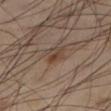{"biopsy_status": "not biopsied; imaged during a skin examination", "automated_metrics": {"area_mm2_approx": 2.5, "shape_asymmetry": 0.35, "nevus_likeness_0_100": 75, "lesion_detection_confidence_0_100": 100}, "image": {"source": "total-body photography crop", "field_of_view_mm": 15}, "patient": {"sex": "male", "age_approx": 55}, "lesion_size": {"long_diameter_mm_approx": 2.5}, "site": "leg", "lighting": "cross-polarized"}